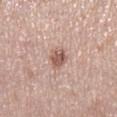A female patient aged around 40. A lesion tile, about 15 mm wide, cut from a 3D total-body photograph. The lesion is on the right lower leg.